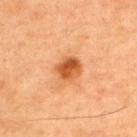This lesion was catalogued during total-body skin photography and was not selected for biopsy.
A male subject in their mid- to late 40s.
A 15 mm crop from a total-body photograph taken for skin-cancer surveillance.
Imaged with cross-polarized lighting.
From the upper back.
Measured at roughly 3 mm in maximum diameter.